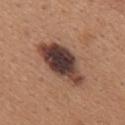Captured during whole-body skin photography for melanoma surveillance; the lesion was not biopsied.
This image is a 15 mm lesion crop taken from a total-body photograph.
The tile uses white-light illumination.
The subject is a female aged around 30.
Approximately 7 mm at its widest.
The lesion is on the back.